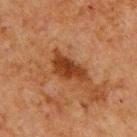workup: total-body-photography surveillance lesion; no biopsy
subject: male, aged around 60
automated metrics: roughly 10 lightness units darker than nearby skin and a lesion-to-skin contrast of about 10 (normalized; higher = more distinct); a lesion-detection confidence of about 100/100
image: 15 mm crop, total-body photography
lighting: cross-polarized illumination
anatomic site: the upper back